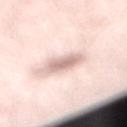Case summary:
– imaging modality · ~15 mm tile from a whole-body skin photo
– lighting · white-light illumination
– subject · female, aged 43–47
– image-analysis metrics · an area of roughly 6.5 mm² and a symmetry-axis asymmetry near 0.2; a border-irregularity rating of about 2/10 and radial color variation of about 1
– lesion diameter · ≈3 mm
– body site · the right lower leg